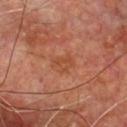Q: Was a biopsy performed?
A: catalogued during a skin exam; not biopsied
Q: Lesion location?
A: the chest
Q: What kind of image is this?
A: ~15 mm crop, total-body skin-cancer survey
Q: Patient demographics?
A: male, in their 60s